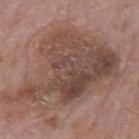Q: What is the lesion's diameter?
A: about 13.5 mm
Q: Who is the patient?
A: male, roughly 75 years of age
Q: What is the anatomic site?
A: the back
Q: Illumination type?
A: white-light
Q: What did automated image analysis measure?
A: a lesion area of about 65 mm², an outline eccentricity of about 0.75 (0 = round, 1 = elongated), and a symmetry-axis asymmetry near 0.35; a border-irregularity index near 6.5/10, a color-variation rating of about 6.5/10, and radial color variation of about 2
Q: What kind of image is this?
A: ~15 mm tile from a whole-body skin photo